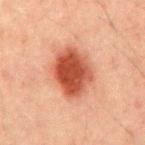Impression:
This lesion was catalogued during total-body skin photography and was not selected for biopsy.
Background:
The total-body-photography lesion software estimated a lesion area of about 19 mm², a shape eccentricity near 0.65, and a symmetry-axis asymmetry near 0.15. And it measured radial color variation of about 1.5. The lesion is located on the mid back. About 5.5 mm across. The patient is a male about 50 years old. The tile uses cross-polarized illumination. A lesion tile, about 15 mm wide, cut from a 3D total-body photograph.